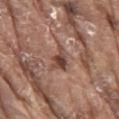<lesion>
<biopsy_status>not biopsied; imaged during a skin examination</biopsy_status>
<lesion_size>
  <long_diameter_mm_approx>3.5</long_diameter_mm_approx>
</lesion_size>
<patient>
  <sex>male</sex>
  <age_approx>80</age_approx>
</patient>
<site>mid back</site>
<image>
  <source>total-body photography crop</source>
  <field_of_view_mm>15</field_of_view_mm>
</image>
<lighting>white-light</lighting>
</lesion>